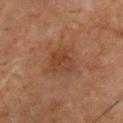biopsy status — total-body-photography surveillance lesion; no biopsy
tile lighting — cross-polarized
image source — 15 mm crop, total-body photography
subject — male, aged around 55
anatomic site — the chest
lesion size — ≈3 mm
TBP lesion metrics — a footprint of about 5.5 mm² and an outline eccentricity of about 0.5 (0 = round, 1 = elongated); border irregularity of about 4 on a 0–10 scale, a within-lesion color-variation index near 2.5/10, and radial color variation of about 1; a classifier nevus-likeness of about 5/100 and a lesion-detection confidence of about 100/100